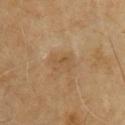* workup · total-body-photography surveillance lesion; no biopsy
* subject · male, approximately 70 years of age
* image · 15 mm crop, total-body photography
* site · the chest
* diameter · about 3 mm